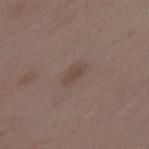notes=total-body-photography surveillance lesion; no biopsy
lighting=white-light
acquisition=total-body-photography crop, ~15 mm field of view
patient=female, aged 33 to 37
location=the back
lesion size=≈3 mm